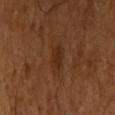| key | value |
|---|---|
| follow-up | total-body-photography surveillance lesion; no biopsy |
| location | the right upper arm |
| subject | male, aged around 50 |
| image source | total-body-photography crop, ~15 mm field of view |
| diameter | ~3 mm (longest diameter) |